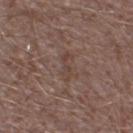biopsy_status: not biopsied; imaged during a skin examination
site: left lower leg
image:
  source: total-body photography crop
  field_of_view_mm: 15
patient:
  sex: male
  age_approx: 45